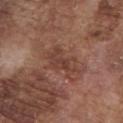Clinical impression:
Imaged during a routine full-body skin examination; the lesion was not biopsied and no histopathology is available.
Context:
A 15 mm close-up extracted from a 3D total-body photography capture. The total-body-photography lesion software estimated an area of roughly 8 mm², a shape eccentricity near 0.8, and a symmetry-axis asymmetry near 0.4. It also reported border irregularity of about 5 on a 0–10 scale, a color-variation rating of about 3/10, and peripheral color asymmetry of about 1. And it measured an automated nevus-likeness rating near 0 out of 100. The patient is a male aged 73 to 77. The recorded lesion diameter is about 4.5 mm. On the chest. This is a white-light tile.